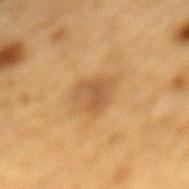No biopsy was performed on this lesion — it was imaged during a full skin examination and was not determined to be concerning. This is a cross-polarized tile. A region of skin cropped from a whole-body photographic capture, roughly 15 mm wide. From the mid back. The patient is a male approximately 85 years of age or older. About 3.5 mm across.